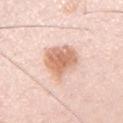Recorded during total-body skin imaging; not selected for excision or biopsy.
Approximately 5 mm at its widest.
The lesion is located on the right upper arm.
Automated tile analysis of the lesion measured a lesion color around L≈70 a*≈22 b*≈31 in CIELAB, roughly 13 lightness units darker than nearby skin, and a normalized lesion–skin contrast near 8.5. And it measured a detector confidence of about 100 out of 100 that the crop contains a lesion.
A male subject, in their mid-30s.
The tile uses white-light illumination.
A 15 mm close-up tile from a total-body photography series done for melanoma screening.